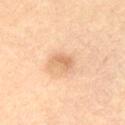biopsy status = total-body-photography surveillance lesion; no biopsy | tile lighting = cross-polarized illumination | location = the chest | patient = male, approximately 65 years of age | image = total-body-photography crop, ~15 mm field of view | lesion size = ≈2.5 mm.